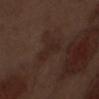Impression: This lesion was catalogued during total-body skin photography and was not selected for biopsy. Background: Automated image analysis of the tile measured a nevus-likeness score of about 0/100 and a detector confidence of about 100 out of 100 that the crop contains a lesion. A male subject, about 70 years old. A 15 mm close-up extracted from a 3D total-body photography capture. The lesion is on the abdomen. Longest diameter approximately 3.5 mm.